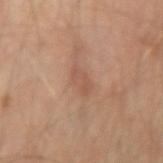No biopsy was performed on this lesion — it was imaged during a full skin examination and was not determined to be concerning.
Located on the right forearm.
The subject is about 55 years old.
A roughly 15 mm field-of-view crop from a total-body skin photograph.
Imaged with cross-polarized lighting.